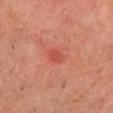follow-up: no biopsy performed (imaged during a skin exam) | acquisition: ~15 mm tile from a whole-body skin photo | subject: male, aged approximately 60 | location: the head or neck.